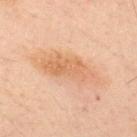  biopsy_status: not biopsied; imaged during a skin examination
  site: back
  automated_metrics:
    cielab_L: 59
    cielab_a: 20
    cielab_b: 34
    vs_skin_darker_L: 8.0
    vs_skin_contrast_norm: 6.5
    border_irregularity_0_10: 4.0
    color_variation_0_10: 3.5
    peripheral_color_asymmetry: 1.0
    nevus_likeness_0_100: 15
    lesion_detection_confidence_0_100: 100
  image:
    source: total-body photography crop
    field_of_view_mm: 15
  patient:
    sex: male
    age_approx: 50
  lesion_size:
    long_diameter_mm_approx: 7.0
  lighting: cross-polarized